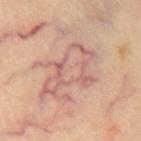biopsy status: total-body-photography surveillance lesion; no biopsy | imaging modality: 15 mm crop, total-body photography | anatomic site: the chest.